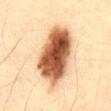Assessment:
Recorded during total-body skin imaging; not selected for excision or biopsy.
Acquisition and patient details:
On the front of the torso. A close-up tile cropped from a whole-body skin photograph, about 15 mm across. A male subject, aged around 35.